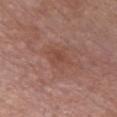Imaged during a routine full-body skin examination; the lesion was not biopsied and no histopathology is available. Longest diameter approximately 3.5 mm. Imaged with white-light lighting. On the chest. A male patient aged 58 to 62. A lesion tile, about 15 mm wide, cut from a 3D total-body photograph.